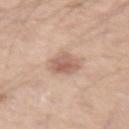tile lighting=white-light illumination | patient=male, aged around 45 | location=the left upper arm | lesion diameter=≈3 mm | acquisition=total-body-photography crop, ~15 mm field of view.